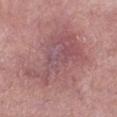Q: Is there a histopathology result?
A: catalogued during a skin exam; not biopsied
Q: What did automated image analysis measure?
A: an outline eccentricity of about 0.7 (0 = round, 1 = elongated); border irregularity of about 6 on a 0–10 scale, a color-variation rating of about 5.5/10, and radial color variation of about 1.5
Q: Lesion size?
A: ≈9 mm
Q: Where on the body is the lesion?
A: the left lower leg
Q: Who is the patient?
A: female, aged 58–62
Q: What lighting was used for the tile?
A: white-light
Q: How was this image acquired?
A: ~15 mm tile from a whole-body skin photo